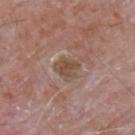Clinical impression:
Imaged during a routine full-body skin examination; the lesion was not biopsied and no histopathology is available.
Clinical summary:
About 3 mm across. The lesion is on the upper back. Automated image analysis of the tile measured a mean CIELAB color near L≈48 a*≈16 b*≈27, roughly 8 lightness units darker than nearby skin, and a normalized border contrast of about 7.5. It also reported a border-irregularity index near 4/10, a color-variation rating of about 2.5/10, and radial color variation of about 0.5. A male subject aged around 65. Captured under white-light illumination. A region of skin cropped from a whole-body photographic capture, roughly 15 mm wide.